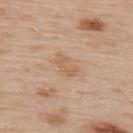| feature | finding |
|---|---|
| follow-up | imaged on a skin check; not biopsied |
| image-analysis metrics | a lesion area of about 4 mm², an outline eccentricity of about 0.85 (0 = round, 1 = elongated), and a shape-asymmetry score of about 0.3 (0 = symmetric); a lesion color around L≈60 a*≈18 b*≈33 in CIELAB, roughly 7 lightness units darker than nearby skin, and a normalized border contrast of about 5.5; border irregularity of about 3.5 on a 0–10 scale, a within-lesion color-variation index near 1/10, and peripheral color asymmetry of about 0.5; a classifier nevus-likeness of about 0/100 and a detector confidence of about 100 out of 100 that the crop contains a lesion |
| imaging modality | total-body-photography crop, ~15 mm field of view |
| tile lighting | white-light illumination |
| lesion diameter | about 3 mm |
| subject | male, aged 53–57 |
| site | the upper back |